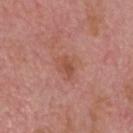Captured during whole-body skin photography for melanoma surveillance; the lesion was not biopsied. A male subject aged around 75. Located on the head or neck. A close-up tile cropped from a whole-body skin photograph, about 15 mm across. Captured under white-light illumination.